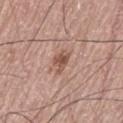  biopsy_status: not biopsied; imaged during a skin examination